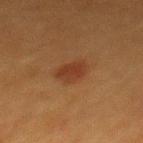Clinical impression: The lesion was tiled from a total-body skin photograph and was not biopsied. Context: The subject is a female roughly 40 years of age. Approximately 3 mm at its widest. The lesion is on the mid back. A 15 mm close-up extracted from a 3D total-body photography capture.